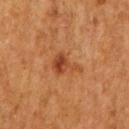The lesion was photographed on a routine skin check and not biopsied; there is no pathology result.
Automated tile analysis of the lesion measured a mean CIELAB color near L≈37 a*≈23 b*≈32, about 8 CIELAB-L* units darker than the surrounding skin, and a normalized lesion–skin contrast near 7.5. It also reported a border-irregularity index near 6.5/10, a color-variation rating of about 3/10, and radial color variation of about 1. It also reported a classifier nevus-likeness of about 80/100.
A close-up tile cropped from a whole-body skin photograph, about 15 mm across.
On the right upper arm.
A female patient, aged 48–52.